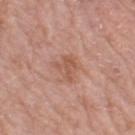- biopsy status: total-body-photography surveillance lesion; no biopsy
- imaging modality: ~15 mm tile from a whole-body skin photo
- illumination: white-light
- diameter: about 2.5 mm
- image-analysis metrics: a footprint of about 5 mm²; a color-variation rating of about 2/10 and peripheral color asymmetry of about 0.5; a nevus-likeness score of about 0/100 and a lesion-detection confidence of about 100/100
- body site: the left thigh
- patient: female, aged 68 to 72